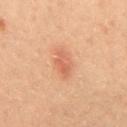Q: What are the patient's age and sex?
A: male, approximately 50 years of age
Q: Automated lesion metrics?
A: a lesion area of about 6 mm², a shape eccentricity near 0.85, and a symmetry-axis asymmetry near 0.3; a classifier nevus-likeness of about 65/100
Q: How was this image acquired?
A: total-body-photography crop, ~15 mm field of view
Q: Lesion location?
A: the front of the torso
Q: What is the lesion's diameter?
A: about 3.5 mm